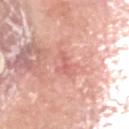Notes:
• workup · imaged on a skin check; not biopsied
• subject · male, aged approximately 65
• size · ≈2.5 mm
• TBP lesion metrics · an area of roughly 2.5 mm², a shape eccentricity near 0.9, and a symmetry-axis asymmetry near 0.6; a lesion-detection confidence of about 95/100
• tile lighting · white-light illumination
• site · the head or neck
• acquisition · total-body-photography crop, ~15 mm field of view